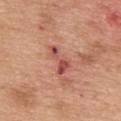The lesion was photographed on a routine skin check and not biopsied; there is no pathology result. The lesion is on the upper back. Captured under white-light illumination. The recorded lesion diameter is about 4 mm. A roughly 15 mm field-of-view crop from a total-body skin photograph. A male patient, approximately 50 years of age.